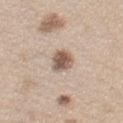This lesion was catalogued during total-body skin photography and was not selected for biopsy. The lesion is on the left upper arm. A 15 mm crop from a total-body photograph taken for skin-cancer surveillance. A female subject aged 43–47.